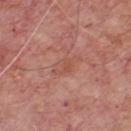{
  "biopsy_status": "not biopsied; imaged during a skin examination"
}